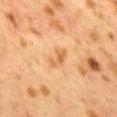workup=no biopsy performed (imaged during a skin exam); patient=female, approximately 40 years of age; illumination=cross-polarized illumination; site=the mid back; image=total-body-photography crop, ~15 mm field of view.